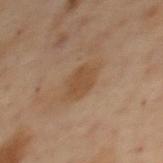follow-up = imaged on a skin check; not biopsied | patient = female, approximately 55 years of age | site = the upper back | size = about 3.5 mm | lighting = cross-polarized illumination | image source = ~15 mm crop, total-body skin-cancer survey.